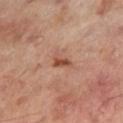image = total-body-photography crop, ~15 mm field of view | automated metrics = an average lesion color of about L≈46 a*≈22 b*≈29 (CIELAB), roughly 9 lightness units darker than nearby skin, and a normalized border contrast of about 7.5; an automated nevus-likeness rating near 60 out of 100 and a detector confidence of about 100 out of 100 that the crop contains a lesion | lighting = cross-polarized | lesion size = about 3 mm | subject = male, aged approximately 60 | anatomic site = the leg.